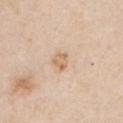The tile uses white-light illumination. Approximately 3.5 mm at its widest. A male subject, aged 63 to 67. The lesion is located on the chest. Cropped from a total-body skin-imaging series; the visible field is about 15 mm.